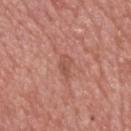Findings:
- follow-up: imaged on a skin check; not biopsied
- subject: male, roughly 60 years of age
- anatomic site: the upper back
- image: total-body-photography crop, ~15 mm field of view